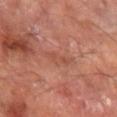biopsy_status: not biopsied; imaged during a skin examination
site: left forearm
lighting: cross-polarized
automated_metrics:
  cielab_L: 48
  cielab_a: 25
  cielab_b: 30
  vs_skin_darker_L: 5.0
  vs_skin_contrast_norm: 4.5
  border_irregularity_0_10: 3.5
  nevus_likeness_0_100: 0
  lesion_detection_confidence_0_100: 100
image:
  source: total-body photography crop
  field_of_view_mm: 15
patient:
  sex: male
  age_approx: 65